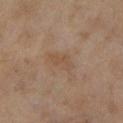This lesion was catalogued during total-body skin photography and was not selected for biopsy.
The tile uses cross-polarized illumination.
About 3.5 mm across.
A female subject, in their 60s.
The lesion is on the left lower leg.
A roughly 15 mm field-of-view crop from a total-body skin photograph.